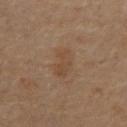workup: imaged on a skin check; not biopsied
location: the abdomen
image: ~15 mm crop, total-body skin-cancer survey
lesion diameter: ≈4 mm
patient: male, in their mid-60s